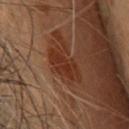The lesion was tiled from a total-body skin photograph and was not biopsied. Automated tile analysis of the lesion measured a footprint of about 10 mm², an outline eccentricity of about 0.75 (0 = round, 1 = elongated), and two-axis asymmetry of about 0.2. The lesion is located on the upper back. The subject is a female aged around 60. Captured under cross-polarized illumination. This image is a 15 mm lesion crop taken from a total-body photograph.